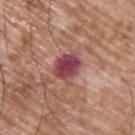• lighting: white-light
• lesion size: ≈3.5 mm
• acquisition: ~15 mm crop, total-body skin-cancer survey
• location: the upper back
• patient: male, approximately 65 years of age